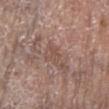biopsy_status: not biopsied; imaged during a skin examination
patient:
  sex: female
  age_approx: 85+
lesion_size:
  long_diameter_mm_approx: 3.0
site: left lower leg
image:
  source: total-body photography crop
  field_of_view_mm: 15